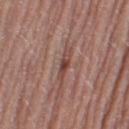The lesion was photographed on a routine skin check and not biopsied; there is no pathology result.
A female patient, about 60 years old.
A lesion tile, about 15 mm wide, cut from a 3D total-body photograph.
The lesion is located on the left thigh.
An algorithmic analysis of the crop reported a border-irregularity rating of about 4/10, a color-variation rating of about 2/10, and radial color variation of about 0.5.